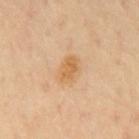notes: catalogued during a skin exam; not biopsied
patient: male, roughly 70 years of age
lesion diameter: ≈3.5 mm
imaging modality: ~15 mm crop, total-body skin-cancer survey
site: the mid back